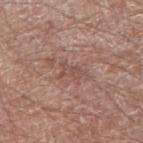Assessment: No biopsy was performed on this lesion — it was imaged during a full skin examination and was not determined to be concerning. Clinical summary: This image is a 15 mm lesion crop taken from a total-body photograph. An algorithmic analysis of the crop reported an eccentricity of roughly 0.85 and two-axis asymmetry of about 0.45. The software also gave border irregularity of about 5 on a 0–10 scale, a within-lesion color-variation index near 1.5/10, and a peripheral color-asymmetry measure near 0.5. The software also gave a classifier nevus-likeness of about 0/100 and lesion-presence confidence of about 55/100. The subject is a male roughly 65 years of age. Captured under white-light illumination. Located on the right upper arm.